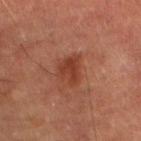{"biopsy_status": "not biopsied; imaged during a skin examination", "lesion_size": {"long_diameter_mm_approx": 3.5}, "image": {"source": "total-body photography crop", "field_of_view_mm": 15}, "site": "left lower leg", "patient": {"sex": "male", "age_approx": "85+"}, "lighting": "cross-polarized"}